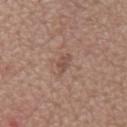| key | value |
|---|---|
| notes | imaged on a skin check; not biopsied |
| lesion size | about 2.5 mm |
| location | the mid back |
| patient | male, in their mid-60s |
| image | ~15 mm crop, total-body skin-cancer survey |
| lighting | white-light |